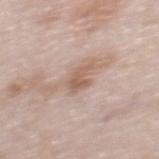Assessment: This lesion was catalogued during total-body skin photography and was not selected for biopsy. Clinical summary: The tile uses white-light illumination. A female subject in their mid-60s. The lesion is on the back. A 15 mm close-up tile from a total-body photography series done for melanoma screening.